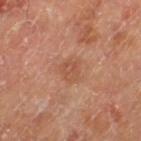follow-up — catalogued during a skin exam; not biopsied | diameter — ≈2.5 mm | image — ~15 mm tile from a whole-body skin photo | subject — male, about 70 years old | location — the leg.